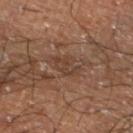Part of a total-body skin-imaging series; this lesion was reviewed on a skin check and was not flagged for biopsy.
A region of skin cropped from a whole-body photographic capture, roughly 15 mm wide.
A male patient aged 58 to 62.
The tile uses cross-polarized illumination.
The lesion is located on the left lower leg.